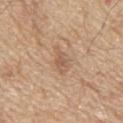workup — catalogued during a skin exam; not biopsied | tile lighting — white-light | patient — male, aged 68 to 72 | lesion size — ~3.5 mm (longest diameter) | anatomic site — the upper back | acquisition — 15 mm crop, total-body photography.